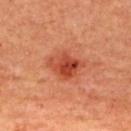Findings:
* biopsy diagnosis · a superficial basal cell carcinoma